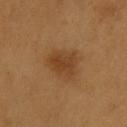notes = no biopsy performed (imaged during a skin exam).